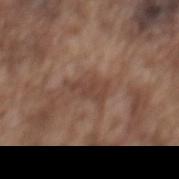Clinical impression:
No biopsy was performed on this lesion — it was imaged during a full skin examination and was not determined to be concerning.
Acquisition and patient details:
Captured under white-light illumination. Approximately 4 mm at its widest. The total-body-photography lesion software estimated a footprint of about 5.5 mm², an eccentricity of roughly 0.85, and two-axis asymmetry of about 0.35. It also reported a mean CIELAB color near L≈43 a*≈19 b*≈25, about 7 CIELAB-L* units darker than the surrounding skin, and a normalized lesion–skin contrast near 6. The analysis additionally found a color-variation rating of about 2.5/10. A male patient in their mid-70s. A 15 mm crop from a total-body photograph taken for skin-cancer surveillance. From the mid back.